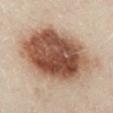Q: Was a biopsy performed?
A: imaged on a skin check; not biopsied
Q: Who is the patient?
A: male, approximately 50 years of age
Q: Automated lesion metrics?
A: a footprint of about 60 mm², an outline eccentricity of about 0.7 (0 = round, 1 = elongated), and a symmetry-axis asymmetry near 0.1; a border-irregularity index near 2/10, a within-lesion color-variation index near 8/10, and a peripheral color-asymmetry measure near 2; an automated nevus-likeness rating near 100 out of 100 and lesion-presence confidence of about 100/100
Q: What lighting was used for the tile?
A: cross-polarized
Q: Lesion location?
A: the left leg
Q: What is the lesion's diameter?
A: ~10.5 mm (longest diameter)
Q: How was this image acquired?
A: ~15 mm tile from a whole-body skin photo